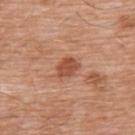Q: Was this lesion biopsied?
A: imaged on a skin check; not biopsied
Q: What are the patient's age and sex?
A: male, in their 60s
Q: What is the imaging modality?
A: 15 mm crop, total-body photography
Q: Illumination type?
A: white-light illumination
Q: Lesion location?
A: the front of the torso